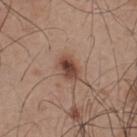Clinical impression:
This lesion was catalogued during total-body skin photography and was not selected for biopsy.
Image and clinical context:
An algorithmic analysis of the crop reported a footprint of about 5.5 mm², an outline eccentricity of about 0.75 (0 = round, 1 = elongated), and two-axis asymmetry of about 0.25. It also reported border irregularity of about 2.5 on a 0–10 scale, a color-variation rating of about 5.5/10, and radial color variation of about 2. Captured under white-light illumination. Measured at roughly 3 mm in maximum diameter. A male patient aged around 50. A lesion tile, about 15 mm wide, cut from a 3D total-body photograph. Located on the upper back.